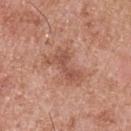follow-up = total-body-photography surveillance lesion; no biopsy | subject = male, aged around 55 | imaging modality = ~15 mm crop, total-body skin-cancer survey | body site = the back.